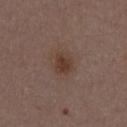This lesion was catalogued during total-body skin photography and was not selected for biopsy. The lesion is located on the abdomen. A male patient, in their 50s. The lesion's longest dimension is about 2.5 mm. A 15 mm close-up extracted from a 3D total-body photography capture.